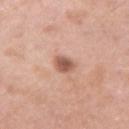patient: female, aged around 40
tile lighting: white-light illumination
image: ~15 mm crop, total-body skin-cancer survey
image-analysis metrics: an area of roughly 4 mm² and two-axis asymmetry of about 0.25; a lesion color around L≈56 a*≈23 b*≈28 in CIELAB, roughly 14 lightness units darker than nearby skin, and a normalized lesion–skin contrast near 9
anatomic site: the right upper arm
size: ~2.5 mm (longest diameter)